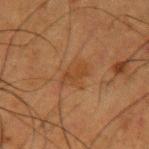<record>
  <biopsy_status>not biopsied; imaged during a skin examination</biopsy_status>
  <site>left upper arm</site>
  <image>
    <source>total-body photography crop</source>
    <field_of_view_mm>15</field_of_view_mm>
  </image>
  <lesion_size>
    <long_diameter_mm_approx>3.5</long_diameter_mm_approx>
  </lesion_size>
  <lighting>cross-polarized</lighting>
  <patient>
    <sex>male</sex>
    <age_approx>50</age_approx>
  </patient>
</record>